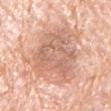Assessment:
The lesion was photographed on a routine skin check and not biopsied; there is no pathology result.
Context:
This image is a 15 mm lesion crop taken from a total-body photograph. Automated image analysis of the tile measured a lesion area of about 32 mm² and an eccentricity of roughly 0.8. The analysis additionally found an average lesion color of about L≈65 a*≈22 b*≈30 (CIELAB), roughly 11 lightness units darker than nearby skin, and a normalized border contrast of about 6.5. The analysis additionally found a within-lesion color-variation index near 4.5/10 and radial color variation of about 1.5. The lesion's longest dimension is about 9 mm. The lesion is on the arm. A male subject, roughly 80 years of age.